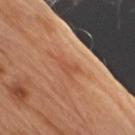Clinical impression: No biopsy was performed on this lesion — it was imaged during a full skin examination and was not determined to be concerning. Context: The total-body-photography lesion software estimated a footprint of about 4 mm², an outline eccentricity of about 0.85 (0 = round, 1 = elongated), and two-axis asymmetry of about 0.55. It also reported lesion-presence confidence of about 90/100. A roughly 15 mm field-of-view crop from a total-body skin photograph. Imaged with white-light lighting. On the arm. A male patient, in their 70s.